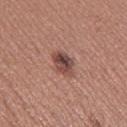Notes:
- notes · catalogued during a skin exam; not biopsied
- subject · female, aged approximately 30
- location · the left thigh
- image source · ~15 mm crop, total-body skin-cancer survey